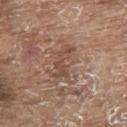This lesion was catalogued during total-body skin photography and was not selected for biopsy. The subject is a male aged around 80. A lesion tile, about 15 mm wide, cut from a 3D total-body photograph. This is a white-light tile. On the upper back. The total-body-photography lesion software estimated an area of roughly 4.5 mm², an eccentricity of roughly 0.9, and two-axis asymmetry of about 0.5. It also reported a mean CIELAB color near L≈47 a*≈19 b*≈26, a lesion–skin lightness drop of about 8, and a normalized border contrast of about 6. And it measured a border-irregularity index near 7.5/10, a within-lesion color-variation index near 0/10, and radial color variation of about 0. The software also gave a nevus-likeness score of about 0/100 and lesion-presence confidence of about 90/100. Longest diameter approximately 4 mm.